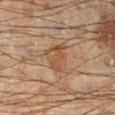Captured under cross-polarized illumination. This image is a 15 mm lesion crop taken from a total-body photograph. From the left leg. A male patient, in their 60s.